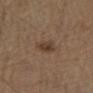Clinical summary: The lesion-visualizer software estimated an area of roughly 5 mm², an eccentricity of roughly 0.4, and two-axis asymmetry of about 0.25. The analysis additionally found a mean CIELAB color near L≈39 a*≈16 b*≈27, about 8 CIELAB-L* units darker than the surrounding skin, and a normalized lesion–skin contrast near 7. It also reported a within-lesion color-variation index near 3.5/10 and peripheral color asymmetry of about 1.5. Imaged with white-light lighting. A male patient, in their mid-70s. The lesion is located on the leg. A roughly 15 mm field-of-view crop from a total-body skin photograph. Approximately 2.5 mm at its widest.